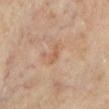Q: Is there a histopathology result?
A: total-body-photography surveillance lesion; no biopsy
Q: What lighting was used for the tile?
A: cross-polarized illumination
Q: What is the imaging modality?
A: 15 mm crop, total-body photography
Q: What are the patient's age and sex?
A: female, aged approximately 70
Q: Lesion location?
A: the left lower leg
Q: Lesion size?
A: ≈2.5 mm